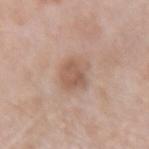| field | value |
|---|---|
| follow-up | no biopsy performed (imaged during a skin exam) |
| automated metrics | a border-irregularity rating of about 2/10, internal color variation of about 3.5 on a 0–10 scale, and a peripheral color-asymmetry measure near 1 |
| anatomic site | the right forearm |
| image | ~15 mm tile from a whole-body skin photo |
| patient | female, in their mid-60s |